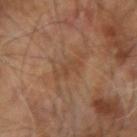Impression: Captured during whole-body skin photography for melanoma surveillance; the lesion was not biopsied. Background: Longest diameter approximately 3 mm. On the right arm. A close-up tile cropped from a whole-body skin photograph, about 15 mm across. The patient is a male aged 58 to 62.